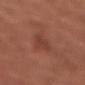This lesion was catalogued during total-body skin photography and was not selected for biopsy. Longest diameter approximately 3 mm. A female patient, roughly 65 years of age. A 15 mm close-up tile from a total-body photography series done for melanoma screening. The lesion-visualizer software estimated an average lesion color of about L≈40 a*≈24 b*≈27 (CIELAB), roughly 6 lightness units darker than nearby skin, and a normalized border contrast of about 5.5. It also reported a border-irregularity index near 3/10, internal color variation of about 1 on a 0–10 scale, and radial color variation of about 0.5. The analysis additionally found a nevus-likeness score of about 0/100 and lesion-presence confidence of about 85/100. From the left forearm. The tile uses white-light illumination.